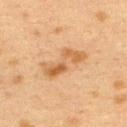Part of a total-body skin-imaging series; this lesion was reviewed on a skin check and was not flagged for biopsy.
Automated tile analysis of the lesion measured an average lesion color of about L≈50 a*≈19 b*≈35 (CIELAB) and a normalized border contrast of about 7.5. It also reported a nevus-likeness score of about 5/100 and a detector confidence of about 100 out of 100 that the crop contains a lesion.
A lesion tile, about 15 mm wide, cut from a 3D total-body photograph.
On the back.
Captured under cross-polarized illumination.
The subject is a female in their 40s.
The recorded lesion diameter is about 5.5 mm.